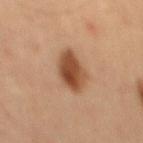The lesion was photographed on a routine skin check and not biopsied; there is no pathology result.
Captured under cross-polarized illumination.
This image is a 15 mm lesion crop taken from a total-body photograph.
The total-body-photography lesion software estimated an area of roughly 11 mm², a shape eccentricity near 0.85, and a symmetry-axis asymmetry near 0.15. The software also gave a lesion color around L≈47 a*≈22 b*≈33 in CIELAB, a lesion–skin lightness drop of about 14, and a normalized border contrast of about 10. It also reported a lesion-detection confidence of about 100/100.
A male patient, in their 60s.
The lesion is located on the mid back.